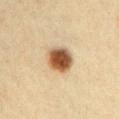follow-up: no biopsy performed (imaged during a skin exam) | TBP lesion metrics: a footprint of about 9 mm², an outline eccentricity of about 0.4 (0 = round, 1 = elongated), and a shape-asymmetry score of about 0.1 (0 = symmetric); an average lesion color of about L≈48 a*≈18 b*≈33 (CIELAB), about 19 CIELAB-L* units darker than the surrounding skin, and a normalized lesion–skin contrast near 13; border irregularity of about 1 on a 0–10 scale | patient: male, about 40 years old | tile lighting: cross-polarized illumination | location: the left upper arm | size: about 3.5 mm | image: 15 mm crop, total-body photography.